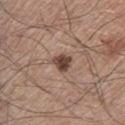No biopsy was performed on this lesion — it was imaged during a full skin examination and was not determined to be concerning. Cropped from a whole-body photographic skin survey; the tile spans about 15 mm. The subject is a male in their mid-60s. Located on the leg.